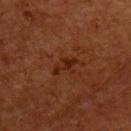This lesion was catalogued during total-body skin photography and was not selected for biopsy.
Automated tile analysis of the lesion measured an area of roughly 3.5 mm², an eccentricity of roughly 0.9, and a symmetry-axis asymmetry near 0.4. And it measured roughly 7 lightness units darker than nearby skin and a normalized lesion–skin contrast near 7.5. The analysis additionally found border irregularity of about 5.5 on a 0–10 scale and radial color variation of about 0. The software also gave an automated nevus-likeness rating near 0 out of 100.
Approximately 3.5 mm at its widest.
The patient is a male in their mid- to late 50s.
On the upper back.
A 15 mm close-up tile from a total-body photography series done for melanoma screening.